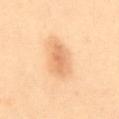Imaged during a routine full-body skin examination; the lesion was not biopsied and no histopathology is available. A region of skin cropped from a whole-body photographic capture, roughly 15 mm wide. On the abdomen. The lesion's longest dimension is about 4 mm. The tile uses cross-polarized illumination. The subject is a female aged 38 to 42.